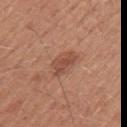Assessment: Part of a total-body skin-imaging series; this lesion was reviewed on a skin check and was not flagged for biopsy. Context: The lesion is on the left upper arm. Cropped from a whole-body photographic skin survey; the tile spans about 15 mm. Longest diameter approximately 4 mm. The lesion-visualizer software estimated a footprint of about 5.5 mm² and a shape eccentricity near 0.85. The analysis additionally found border irregularity of about 4 on a 0–10 scale, internal color variation of about 2 on a 0–10 scale, and radial color variation of about 0.5. And it measured lesion-presence confidence of about 100/100. Imaged with white-light lighting. A male patient aged 28–32.